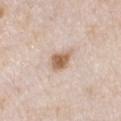Assessment:
This lesion was catalogued during total-body skin photography and was not selected for biopsy.
Acquisition and patient details:
A female patient, aged approximately 45. The tile uses white-light illumination. The lesion is located on the chest. A 15 mm close-up tile from a total-body photography series done for melanoma screening. Approximately 3.5 mm at its widest. Automated image analysis of the tile measured a footprint of about 5.5 mm², an outline eccentricity of about 0.75 (0 = round, 1 = elongated), and two-axis asymmetry of about 0.3. The software also gave a lesion color around L≈62 a*≈17 b*≈31 in CIELAB and a lesion-to-skin contrast of about 9.5 (normalized; higher = more distinct).